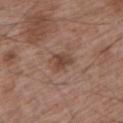notes: catalogued during a skin exam; not biopsied
acquisition: 15 mm crop, total-body photography
TBP lesion metrics: an automated nevus-likeness rating near 15 out of 100 and lesion-presence confidence of about 100/100
site: the left upper arm
patient: male, aged around 65
lighting: white-light illumination
lesion size: ~3 mm (longest diameter)